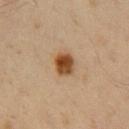Context:
A male patient aged around 60. A lesion tile, about 15 mm wide, cut from a 3D total-body photograph. Imaged with cross-polarized lighting. Approximately 3 mm at its widest. Located on the chest. An algorithmic analysis of the crop reported a classifier nevus-likeness of about 100/100 and lesion-presence confidence of about 100/100.